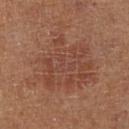workup — catalogued during a skin exam; not biopsied
anatomic site — the right lower leg
image source — 15 mm crop, total-body photography
patient — female